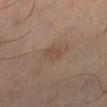Assessment: No biopsy was performed on this lesion — it was imaged during a full skin examination and was not determined to be concerning. Clinical summary: A male patient, in their mid-60s. On the right lower leg. Captured under cross-polarized illumination. Approximately 2.5 mm at its widest. This image is a 15 mm lesion crop taken from a total-body photograph.